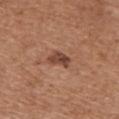The lesion is on the back. This is a white-light tile. A male patient aged approximately 70. A roughly 15 mm field-of-view crop from a total-body skin photograph. An algorithmic analysis of the crop reported an area of roughly 4 mm², a shape eccentricity near 0.8, and a symmetry-axis asymmetry near 0.3. It also reported a lesion–skin lightness drop of about 12 and a lesion-to-skin contrast of about 9 (normalized; higher = more distinct).